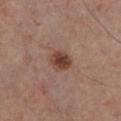Clinical impression: The lesion was tiled from a total-body skin photograph and was not biopsied. Image and clinical context: Automated image analysis of the tile measured a footprint of about 5.5 mm², an outline eccentricity of about 0.6 (0 = round, 1 = elongated), and two-axis asymmetry of about 0.2. And it measured a color-variation rating of about 3.5/10. And it measured a detector confidence of about 100 out of 100 that the crop contains a lesion. A region of skin cropped from a whole-body photographic capture, roughly 15 mm wide. This is a white-light tile. A male subject, aged around 55. The lesion is located on the chest. About 3 mm across.